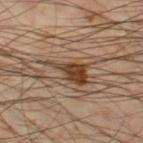{
  "biopsy_status": "not biopsied; imaged during a skin examination",
  "site": "left thigh",
  "image": {
    "source": "total-body photography crop",
    "field_of_view_mm": 15
  },
  "patient": {
    "sex": "male",
    "age_approx": 50
  },
  "lighting": "cross-polarized",
  "lesion_size": {
    "long_diameter_mm_approx": 5.0
  }
}